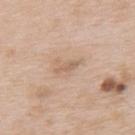biopsy status: no biopsy performed (imaged during a skin exam)
image source: ~15 mm crop, total-body skin-cancer survey
subject: male, roughly 65 years of age
body site: the back